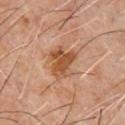Assessment: No biopsy was performed on this lesion — it was imaged during a full skin examination and was not determined to be concerning. Context: Located on the front of the torso. Automated image analysis of the tile measured a classifier nevus-likeness of about 5/100 and a lesion-detection confidence of about 100/100. A male subject, roughly 60 years of age. Captured under cross-polarized illumination. Cropped from a total-body skin-imaging series; the visible field is about 15 mm.